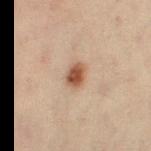  site: leg
  lighting: cross-polarized
  lesion_size:
    long_diameter_mm_approx: 3.0
  patient:
    sex: female
    age_approx: 45
  image:
    source: total-body photography crop
    field_of_view_mm: 15